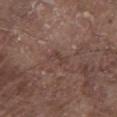Q: Was this lesion biopsied?
A: imaged on a skin check; not biopsied
Q: Who is the patient?
A: male, aged 78 to 82
Q: Lesion location?
A: the chest
Q: How was this image acquired?
A: total-body-photography crop, ~15 mm field of view